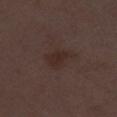biopsy status: imaged on a skin check; not biopsied | image-analysis metrics: an outline eccentricity of about 0.7 (0 = round, 1 = elongated) and a shape-asymmetry score of about 0.35 (0 = symmetric); a normalized border contrast of about 6.5; a border-irregularity index near 3.5/10 and a color-variation rating of about 2/10 | patient: male, aged 48–52 | image source: ~15 mm tile from a whole-body skin photo | illumination: white-light | diameter: ≈3.5 mm.